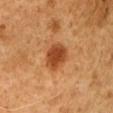Clinical impression: Part of a total-body skin-imaging series; this lesion was reviewed on a skin check and was not flagged for biopsy. Background: The recorded lesion diameter is about 3.5 mm. The lesion-visualizer software estimated a lesion area of about 8.5 mm² and a shape-asymmetry score of about 0.15 (0 = symmetric). The software also gave a within-lesion color-variation index near 3/10 and peripheral color asymmetry of about 1. On the left upper arm. A 15 mm crop from a total-body photograph taken for skin-cancer surveillance. The tile uses cross-polarized illumination. The patient is a male about 50 years old.